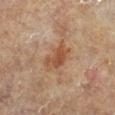Case summary:
* notes: catalogued during a skin exam; not biopsied
* body site: the right lower leg
* patient: male, aged 83 or over
* image source: 15 mm crop, total-body photography
* illumination: cross-polarized illumination